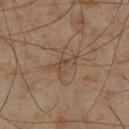The lesion was photographed on a routine skin check and not biopsied; there is no pathology result. A male patient, approximately 45 years of age. On the right lower leg. Approximately 4 mm at its widest. A close-up tile cropped from a whole-body skin photograph, about 15 mm across. Captured under cross-polarized illumination.